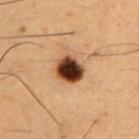Impression:
Part of a total-body skin-imaging series; this lesion was reviewed on a skin check and was not flagged for biopsy.
Acquisition and patient details:
The total-body-photography lesion software estimated an area of roughly 7 mm², an outline eccentricity of about 0.5 (0 = round, 1 = elongated), and a symmetry-axis asymmetry near 0.15. It also reported a lesion color around L≈29 a*≈19 b*≈26 in CIELAB. The analysis additionally found a border-irregularity rating of about 1.5/10, internal color variation of about 6 on a 0–10 scale, and a peripheral color-asymmetry measure near 1.5. The tile uses cross-polarized illumination. The lesion is located on the chest. The subject is a male aged 48–52. A roughly 15 mm field-of-view crop from a total-body skin photograph.